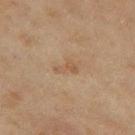Assessment:
The lesion was photographed on a routine skin check and not biopsied; there is no pathology result.
Acquisition and patient details:
The lesion's longest dimension is about 2.5 mm. The lesion is on the left thigh. A female subject aged 58 to 62. The tile uses cross-polarized illumination. A lesion tile, about 15 mm wide, cut from a 3D total-body photograph.